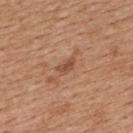The lesion was tiled from a total-body skin photograph and was not biopsied.
Located on the upper back.
The patient is a female about 45 years old.
A 15 mm close-up extracted from a 3D total-body photography capture.
Imaged with white-light lighting.
Longest diameter approximately 2.5 mm.